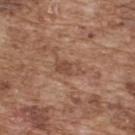{
  "biopsy_status": "not biopsied; imaged during a skin examination",
  "site": "back",
  "image": {
    "source": "total-body photography crop",
    "field_of_view_mm": 15
  },
  "patient": {
    "sex": "male",
    "age_approx": 75
  }
}